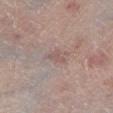The lesion was tiled from a total-body skin photograph and was not biopsied.
On the left lower leg.
The patient is a male approximately 65 years of age.
A lesion tile, about 15 mm wide, cut from a 3D total-body photograph.
Automated tile analysis of the lesion measured a footprint of about 2.5 mm², an outline eccentricity of about 0.8 (0 = round, 1 = elongated), and two-axis asymmetry of about 0.45. And it measured a border-irregularity index near 4.5/10 and a peripheral color-asymmetry measure near 0.
Captured under white-light illumination.